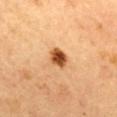biopsy status — imaged on a skin check; not biopsied
location — the back
patient — female, in their 60s
lighting — cross-polarized illumination
imaging modality — 15 mm crop, total-body photography
lesion size — ≈3 mm
image-analysis metrics — an area of roughly 5 mm² and an outline eccentricity of about 0.65 (0 = round, 1 = elongated); roughly 17 lightness units darker than nearby skin and a lesion-to-skin contrast of about 12 (normalized; higher = more distinct); internal color variation of about 4.5 on a 0–10 scale and peripheral color asymmetry of about 1.5; an automated nevus-likeness rating near 100 out of 100 and a lesion-detection confidence of about 100/100